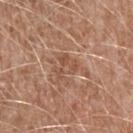This lesion was catalogued during total-body skin photography and was not selected for biopsy. The tile uses white-light illumination. A male patient about 45 years old. The lesion's longest dimension is about 3 mm. A region of skin cropped from a whole-body photographic capture, roughly 15 mm wide. On the left upper arm.